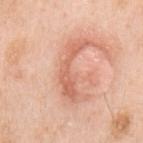{"biopsy_status": "not biopsied; imaged during a skin examination", "image": {"source": "total-body photography crop", "field_of_view_mm": 15}, "automated_metrics": {"area_mm2_approx": 9.5, "eccentricity": 0.95, "shape_asymmetry": 0.7, "nevus_likeness_0_100": 20, "lesion_detection_confidence_0_100": 100}, "site": "arm", "lighting": "white-light", "patient": {"sex": "male", "age_approx": 70}, "lesion_size": {"long_diameter_mm_approx": 7.0}}